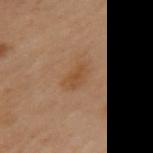Case summary:
– follow-up · imaged on a skin check; not biopsied
– image source · total-body-photography crop, ~15 mm field of view
– image-analysis metrics · peripheral color asymmetry of about 0.5; a classifier nevus-likeness of about 15/100 and a lesion-detection confidence of about 100/100
– lesion diameter · about 3 mm
– subject · female, aged 58–62
– body site · the upper back
– lighting · cross-polarized illumination